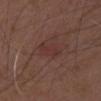workup: total-body-photography surveillance lesion; no biopsy | TBP lesion metrics: an area of roughly 4.5 mm², an outline eccentricity of about 0.9 (0 = round, 1 = elongated), and a symmetry-axis asymmetry near 0.4; border irregularity of about 4.5 on a 0–10 scale, a within-lesion color-variation index near 1/10, and radial color variation of about 0.5; an automated nevus-likeness rating near 0 out of 100 and a detector confidence of about 100 out of 100 that the crop contains a lesion | subject: male, aged around 50 | diameter: ~4 mm (longest diameter) | image: ~15 mm tile from a whole-body skin photo.